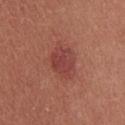biopsy status = imaged on a skin check; not biopsied | site = the chest | lesion size = about 4 mm | lighting = white-light | acquisition = ~15 mm tile from a whole-body skin photo | subject = female, in their mid-30s.